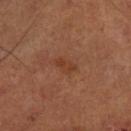Assessment: Imaged during a routine full-body skin examination; the lesion was not biopsied and no histopathology is available. Context: Measured at roughly 3 mm in maximum diameter. A lesion tile, about 15 mm wide, cut from a 3D total-body photograph. A male subject, in their 50s. On the leg.